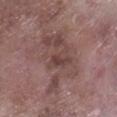follow-up — catalogued during a skin exam; not biopsied
imaging modality — 15 mm crop, total-body photography
anatomic site — the right lower leg
subject — male, roughly 75 years of age